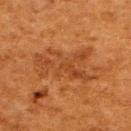Assessment:
The lesion was photographed on a routine skin check and not biopsied; there is no pathology result.
Clinical summary:
The lesion is located on the upper back. Cropped from a whole-body photographic skin survey; the tile spans about 15 mm. The lesion-visualizer software estimated an area of roughly 18 mm² and a shape eccentricity near 0.85. The analysis additionally found a within-lesion color-variation index near 3/10. The analysis additionally found an automated nevus-likeness rating near 0 out of 100 and lesion-presence confidence of about 90/100. Longest diameter approximately 7 mm. The tile uses cross-polarized illumination. A female subject, in their 50s.